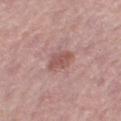{"biopsy_status": "not biopsied; imaged during a skin examination", "patient": {"sex": "female", "age_approx": 50}, "automated_metrics": {"eccentricity": 0.65, "vs_skin_darker_L": 9.0, "vs_skin_contrast_norm": 7.0, "peripheral_color_asymmetry": 1.0, "nevus_likeness_0_100": 65, "lesion_detection_confidence_0_100": 100}, "site": "right thigh", "lesion_size": {"long_diameter_mm_approx": 3.0}, "lighting": "white-light", "image": {"source": "total-body photography crop", "field_of_view_mm": 15}}